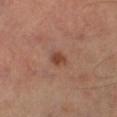biopsy status = imaged on a skin check; not biopsied | subject = male, in their mid- to late 60s | anatomic site = the leg | image = ~15 mm crop, total-body skin-cancer survey.